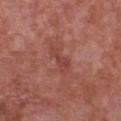Part of a total-body skin-imaging series; this lesion was reviewed on a skin check and was not flagged for biopsy. A 15 mm close-up extracted from a 3D total-body photography capture. Automated image analysis of the tile measured a footprint of about 5 mm² and a shape eccentricity near 0.9. The software also gave a lesion color around L≈44 a*≈29 b*≈26 in CIELAB. The analysis additionally found a nevus-likeness score of about 0/100 and lesion-presence confidence of about 100/100. The lesion is located on the chest. The patient is a male aged approximately 80. Approximately 4 mm at its widest. The tile uses white-light illumination.